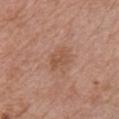{"biopsy_status": "not biopsied; imaged during a skin examination", "site": "chest", "lighting": "white-light", "image": {"source": "total-body photography crop", "field_of_view_mm": 15}, "automated_metrics": {"area_mm2_approx": 4.5, "eccentricity": 0.75, "shape_asymmetry": 0.35, "cielab_L": 52, "cielab_a": 21, "cielab_b": 31, "vs_skin_darker_L": 7.0, "vs_skin_contrast_norm": 6.0, "border_irregularity_0_10": 3.5, "color_variation_0_10": 2.0, "peripheral_color_asymmetry": 1.0, "nevus_likeness_0_100": 0, "lesion_detection_confidence_0_100": 100}, "patient": {"sex": "male", "age_approx": 65}, "lesion_size": {"long_diameter_mm_approx": 3.0}}